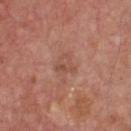Recorded during total-body skin imaging; not selected for excision or biopsy. A male patient, about 75 years old. A close-up tile cropped from a whole-body skin photograph, about 15 mm across. Located on the chest.